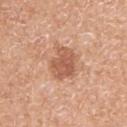• workup — imaged on a skin check; not biopsied
• subject — female, aged approximately 75
• lesion size — about 4 mm
• automated lesion analysis — a mean CIELAB color near L≈57 a*≈24 b*≈33, a lesion–skin lightness drop of about 11, and a normalized border contrast of about 7.5; a nevus-likeness score of about 15/100 and lesion-presence confidence of about 100/100
• image — total-body-photography crop, ~15 mm field of view
• anatomic site — the left upper arm
• lighting — white-light illumination